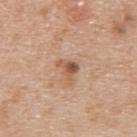Imaged during a routine full-body skin examination; the lesion was not biopsied and no histopathology is available. Located on the upper back. A male subject aged around 65. A lesion tile, about 15 mm wide, cut from a 3D total-body photograph.